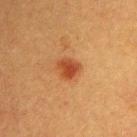Impression: Recorded during total-body skin imaging; not selected for excision or biopsy. Acquisition and patient details: The patient is a male aged 38–42. The lesion is located on the upper back. Cropped from a total-body skin-imaging series; the visible field is about 15 mm.